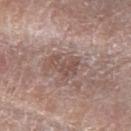<tbp_lesion>
<biopsy_status>not biopsied; imaged during a skin examination</biopsy_status>
<patient>
  <sex>female</sex>
  <age_approx>60</age_approx>
</patient>
<site>right forearm</site>
<image>
  <source>total-body photography crop</source>
  <field_of_view_mm>15</field_of_view_mm>
</image>
<lesion_size>
  <long_diameter_mm_approx>3.5</long_diameter_mm_approx>
</lesion_size>
<lighting>white-light</lighting>
</tbp_lesion>